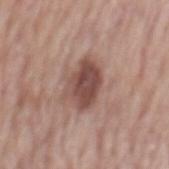Impression: The lesion was tiled from a total-body skin photograph and was not biopsied. Context: About 5 mm across. Located on the mid back. This image is a 15 mm lesion crop taken from a total-body photograph. The total-body-photography lesion software estimated a shape eccentricity near 0.75 and a symmetry-axis asymmetry near 0.25. It also reported about 13 CIELAB-L* units darker than the surrounding skin. It also reported a border-irregularity index near 3/10, internal color variation of about 4 on a 0–10 scale, and peripheral color asymmetry of about 1.5. The analysis additionally found a nevus-likeness score of about 75/100 and lesion-presence confidence of about 100/100. Captured under white-light illumination. A female patient aged 58 to 62.